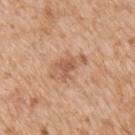acquisition — 15 mm crop, total-body photography | body site — the right upper arm | diameter — ~4.5 mm (longest diameter) | patient — male, aged approximately 65 | image-analysis metrics — a mean CIELAB color near L≈58 a*≈22 b*≈33 and a lesion-to-skin contrast of about 6 (normalized; higher = more distinct); border irregularity of about 5 on a 0–10 scale and radial color variation of about 0.5.